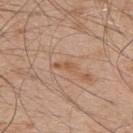notes: catalogued during a skin exam; not biopsied | site: the back | TBP lesion metrics: a lesion area of about 2.5 mm², a shape eccentricity near 0.95, and a shape-asymmetry score of about 0.35 (0 = symmetric); a border-irregularity rating of about 4/10 and a color-variation rating of about 0/10 | lesion diameter: about 3 mm | image source: ~15 mm tile from a whole-body skin photo | patient: male, in their 50s.